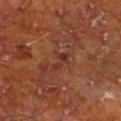Impression:
This lesion was catalogued during total-body skin photography and was not selected for biopsy.
Context:
Imaged with cross-polarized lighting. An algorithmic analysis of the crop reported an area of roughly 3 mm², an eccentricity of roughly 0.9, and a shape-asymmetry score of about 0.45 (0 = symmetric). The analysis additionally found a detector confidence of about 95 out of 100 that the crop contains a lesion. The recorded lesion diameter is about 3 mm. From the left lower leg. A close-up tile cropped from a whole-body skin photograph, about 15 mm across. A male subject approximately 65 years of age.